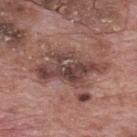The lesion was photographed on a routine skin check and not biopsied; there is no pathology result.
About 8.5 mm across.
Captured under white-light illumination.
The lesion is located on the mid back.
Cropped from a total-body skin-imaging series; the visible field is about 15 mm.
The patient is a male in their 70s.
The total-body-photography lesion software estimated a footprint of about 21 mm² and a shape eccentricity near 0.85. The software also gave a border-irregularity index near 6/10, internal color variation of about 6.5 on a 0–10 scale, and a peripheral color-asymmetry measure near 2.5.